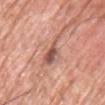Assessment:
Part of a total-body skin-imaging series; this lesion was reviewed on a skin check and was not flagged for biopsy.
Context:
A male patient aged 58 to 62. A 15 mm close-up tile from a total-body photography series done for melanoma screening. The lesion is on the chest. Automated tile analysis of the lesion measured a footprint of about 8.5 mm², an outline eccentricity of about 0.9 (0 = round, 1 = elongated), and a shape-asymmetry score of about 0.45 (0 = symmetric). The analysis additionally found a nevus-likeness score of about 0/100.